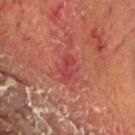This lesion was catalogued during total-body skin photography and was not selected for biopsy. The lesion's longest dimension is about 2.5 mm. The lesion is on the head or neck. The subject is a male roughly 55 years of age. A 15 mm crop from a total-body photograph taken for skin-cancer surveillance.